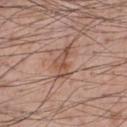Assessment: Captured during whole-body skin photography for melanoma surveillance; the lesion was not biopsied. Background: Cropped from a whole-body photographic skin survey; the tile spans about 15 mm. Imaged with white-light lighting. A male patient, aged around 55. Located on the chest. The recorded lesion diameter is about 4.5 mm. The total-body-photography lesion software estimated a footprint of about 6 mm², a shape eccentricity near 0.9, and a shape-asymmetry score of about 0.5 (0 = symmetric). It also reported a nevus-likeness score of about 0/100.